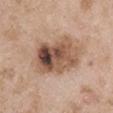notes: imaged on a skin check; not biopsied | location: the right upper arm | subject: male, approximately 50 years of age | illumination: white-light | image: total-body-photography crop, ~15 mm field of view | lesion diameter: ≈7.5 mm.